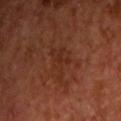Context:
Longest diameter approximately 4 mm. The tile uses cross-polarized illumination. The patient is a male aged 53 to 57. An algorithmic analysis of the crop reported an eccentricity of roughly 0.7 and a shape-asymmetry score of about 0.55 (0 = symmetric). It also reported an average lesion color of about L≈26 a*≈22 b*≈27 (CIELAB) and a normalized lesion–skin contrast near 5. And it measured a border-irregularity index near 6/10, a color-variation rating of about 2/10, and peripheral color asymmetry of about 0.5. A 15 mm close-up tile from a total-body photography series done for melanoma screening. Located on the chest.